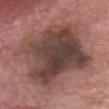{
  "biopsy_status": "not biopsied; imaged during a skin examination",
  "patient": {
    "sex": "male",
    "age_approx": 60
  },
  "site": "head or neck",
  "lesion_size": {
    "long_diameter_mm_approx": 9.0
  },
  "image": {
    "source": "total-body photography crop",
    "field_of_view_mm": 15
  },
  "lighting": "white-light",
  "automated_metrics": {
    "area_mm2_approx": 60.0,
    "eccentricity": 0.45,
    "shape_asymmetry": 0.15,
    "nevus_likeness_0_100": 0,
    "lesion_detection_confidence_0_100": 90
  }
}